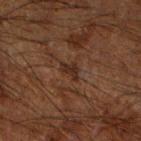- biopsy status · no biopsy performed (imaged during a skin exam)
- TBP lesion metrics · a lesion area of about 2.5 mm² and a shape eccentricity near 0.8; an average lesion color of about L≈21 a*≈15 b*≈21 (CIELAB) and a lesion-to-skin contrast of about 8 (normalized; higher = more distinct)
- anatomic site · the right forearm
- illumination · cross-polarized illumination
- subject · male, aged around 65
- lesion diameter · ~2.5 mm (longest diameter)
- image · ~15 mm crop, total-body skin-cancer survey